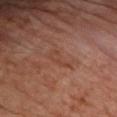follow-up = total-body-photography surveillance lesion; no biopsy
subject = male, aged 63–67
body site = the chest
lesion size = about 3 mm
image source = ~15 mm tile from a whole-body skin photo
image-analysis metrics = a border-irregularity rating of about 6/10 and a color-variation rating of about 0.5/10